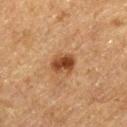Part of a total-body skin-imaging series; this lesion was reviewed on a skin check and was not flagged for biopsy.
Imaged with cross-polarized lighting.
The recorded lesion diameter is about 3 mm.
A lesion tile, about 15 mm wide, cut from a 3D total-body photograph.
A male patient in their mid-70s.
From the left thigh.